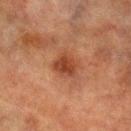Case summary:
• image-analysis metrics: a lesion color around L≈36 a*≈24 b*≈30 in CIELAB, a lesion–skin lightness drop of about 9, and a normalized border contrast of about 8.5; a border-irregularity rating of about 2/10, a color-variation rating of about 2.5/10, and radial color variation of about 0.5; an automated nevus-likeness rating near 55 out of 100 and a lesion-detection confidence of about 100/100
• image: ~15 mm crop, total-body skin-cancer survey
• body site: the left lower leg
• patient: male, approximately 70 years of age